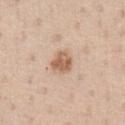Findings:
- follow-up · no biopsy performed (imaged during a skin exam)